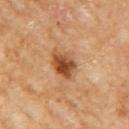  biopsy_status: not biopsied; imaged during a skin examination
  lesion_size:
    long_diameter_mm_approx: 3.5
  lighting: cross-polarized
  image:
    source: total-body photography crop
    field_of_view_mm: 15
  site: right upper arm
  patient:
    sex: female
    age_approx: 60